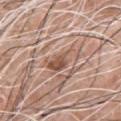The lesion was tiled from a total-body skin photograph and was not biopsied. A roughly 15 mm field-of-view crop from a total-body skin photograph. The subject is a male aged around 60. The lesion is on the chest.